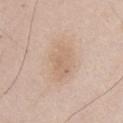Recorded during total-body skin imaging; not selected for excision or biopsy.
A 15 mm crop from a total-body photograph taken for skin-cancer surveillance.
From the front of the torso.
A male patient, approximately 45 years of age.
Approximately 5 mm at its widest.
Imaged with white-light lighting.